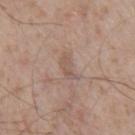Part of a total-body skin-imaging series; this lesion was reviewed on a skin check and was not flagged for biopsy. On the chest. A 15 mm close-up tile from a total-body photography series done for melanoma screening. Captured under white-light illumination. A male patient in their mid- to late 50s. The lesion's longest dimension is about 3.5 mm.